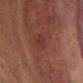Findings:
– notes: imaged on a skin check; not biopsied
– image: ~15 mm tile from a whole-body skin photo
– subject: male, roughly 35 years of age
– anatomic site: the abdomen